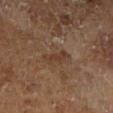Case summary:
– subject · male, aged 63–67
– image source · total-body-photography crop, ~15 mm field of view
– lesion diameter · about 3 mm
– illumination · cross-polarized
– automated lesion analysis · about 6 CIELAB-L* units darker than the surrounding skin and a normalized lesion–skin contrast near 6; border irregularity of about 4.5 on a 0–10 scale, a within-lesion color-variation index near 0/10, and radial color variation of about 0; an automated nevus-likeness rating near 0 out of 100 and lesion-presence confidence of about 100/100